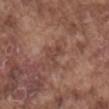| field | value |
|---|---|
| biopsy status | total-body-photography surveillance lesion; no biopsy |
| subject | male, aged approximately 75 |
| diameter | about 3 mm |
| body site | the mid back |
| TBP lesion metrics | an area of roughly 3.5 mm², a shape eccentricity near 0.65, and a shape-asymmetry score of about 0.65 (0 = symmetric); a color-variation rating of about 0.5/10 and peripheral color asymmetry of about 0; a lesion-detection confidence of about 100/100 |
| illumination | white-light illumination |
| imaging modality | total-body-photography crop, ~15 mm field of view |